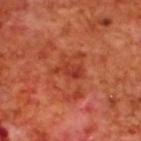  biopsy_status: not biopsied; imaged during a skin examination
  image:
    source: total-body photography crop
    field_of_view_mm: 15
  patient:
    sex: male
    age_approx: 70
  site: upper back
  automated_metrics:
    cielab_L: 39
    cielab_a: 35
    cielab_b: 35
    vs_skin_darker_L: 8.0
    vs_skin_contrast_norm: 6.5